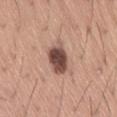biopsy status = no biopsy performed (imaged during a skin exam)
body site = the lower back
illumination = white-light
image-analysis metrics = a mean CIELAB color near L≈48 a*≈20 b*≈24 and about 18 CIELAB-L* units darker than the surrounding skin; border irregularity of about 1.5 on a 0–10 scale, a within-lesion color-variation index near 5/10, and radial color variation of about 1.5; lesion-presence confidence of about 100/100
subject = male, aged 43 to 47
lesion size = about 4 mm
image source = ~15 mm crop, total-body skin-cancer survey